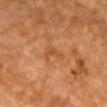{"biopsy_status": "not biopsied; imaged during a skin examination"}